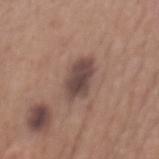Acquisition and patient details: A region of skin cropped from a whole-body photographic capture, roughly 15 mm wide. From the mid back. The patient is a male aged around 65.